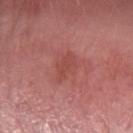Recorded during total-body skin imaging; not selected for excision or biopsy.
The recorded lesion diameter is about 4 mm.
The tile uses white-light illumination.
A female subject, about 60 years old.
Automated image analysis of the tile measured an average lesion color of about L≈48 a*≈29 b*≈26 (CIELAB), a lesion–skin lightness drop of about 6, and a normalized lesion–skin contrast near 5. The software also gave border irregularity of about 3 on a 0–10 scale, a color-variation rating of about 2/10, and peripheral color asymmetry of about 0.5.
Located on the arm.
A region of skin cropped from a whole-body photographic capture, roughly 15 mm wide.